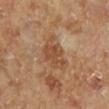Part of a total-body skin-imaging series; this lesion was reviewed on a skin check and was not flagged for biopsy.
The lesion-visualizer software estimated roughly 9 lightness units darker than nearby skin and a normalized lesion–skin contrast near 6.5. And it measured a border-irregularity index near 3.5/10, a within-lesion color-variation index near 2.5/10, and a peripheral color-asymmetry measure near 1.
On the leg.
A 15 mm crop from a total-body photograph taken for skin-cancer surveillance.
The recorded lesion diameter is about 4.5 mm.
The subject is female.
Imaged with cross-polarized lighting.